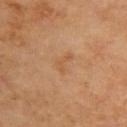Part of a total-body skin-imaging series; this lesion was reviewed on a skin check and was not flagged for biopsy.
A female subject roughly 70 years of age.
This is a cross-polarized tile.
The lesion is located on the upper back.
The lesion's longest dimension is about 2.5 mm.
A 15 mm crop from a total-body photograph taken for skin-cancer surveillance.
Automated tile analysis of the lesion measured an area of roughly 3 mm², an outline eccentricity of about 0.8 (0 = round, 1 = elongated), and two-axis asymmetry of about 0.65.